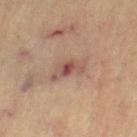<lesion>
  <image>
    <source>total-body photography crop</source>
    <field_of_view_mm>15</field_of_view_mm>
  </image>
  <site>left thigh</site>
  <patient>
    <sex>female</sex>
    <age_approx>65</age_approx>
  </patient>
  <lighting>cross-polarized</lighting>
</lesion>